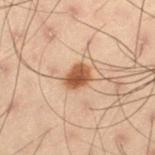Assessment:
Captured during whole-body skin photography for melanoma surveillance; the lesion was not biopsied.
Context:
The patient is a male aged 53–57. The lesion is located on the left thigh. Cropped from a whole-body photographic skin survey; the tile spans about 15 mm. Imaged with cross-polarized lighting.